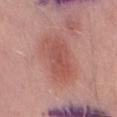Clinical impression: Imaged during a routine full-body skin examination; the lesion was not biopsied and no histopathology is available. Clinical summary: An algorithmic analysis of the crop reported a nevus-likeness score of about 95/100 and lesion-presence confidence of about 100/100. The lesion is on the leg. Longest diameter approximately 6.5 mm. The tile uses white-light illumination. This image is a 15 mm lesion crop taken from a total-body photograph. The subject is a male approximately 50 years of age.